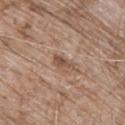body site: the chest
TBP lesion metrics: a footprint of about 3.5 mm², an eccentricity of roughly 0.85, and a symmetry-axis asymmetry near 0.4; a mean CIELAB color near L≈51 a*≈18 b*≈28, roughly 10 lightness units darker than nearby skin, and a lesion-to-skin contrast of about 7 (normalized; higher = more distinct); border irregularity of about 4 on a 0–10 scale, a color-variation rating of about 2.5/10, and a peripheral color-asymmetry measure near 0.5; a classifier nevus-likeness of about 0/100
lesion diameter: ≈3 mm
image source: 15 mm crop, total-body photography
subject: male, aged 63–67
tile lighting: white-light